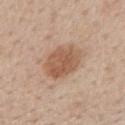Clinical impression: The lesion was photographed on a routine skin check and not biopsied; there is no pathology result. Clinical summary: A roughly 15 mm field-of-view crop from a total-body skin photograph. This is a white-light tile. Located on the mid back. A male patient roughly 60 years of age. The lesion's longest dimension is about 5 mm.